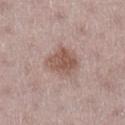Clinical impression:
The lesion was tiled from a total-body skin photograph and was not biopsied.
Image and clinical context:
A female subject aged around 45. From the right lower leg. The tile uses white-light illumination. A 15 mm close-up tile from a total-body photography series done for melanoma screening. Longest diameter approximately 4 mm.